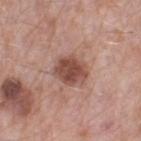biopsy status: catalogued during a skin exam; not biopsied | body site: the right thigh | acquisition: total-body-photography crop, ~15 mm field of view | automated metrics: a lesion area of about 9 mm², an eccentricity of roughly 0.5, and two-axis asymmetry of about 0.15; a color-variation rating of about 3.5/10 and radial color variation of about 1 | subject: male, in their mid- to late 50s.